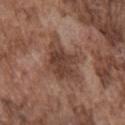The lesion was tiled from a total-body skin photograph and was not biopsied.
A male subject, in their mid-70s.
The lesion is located on the chest.
Measured at roughly 6 mm in maximum diameter.
A close-up tile cropped from a whole-body skin photograph, about 15 mm across.